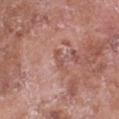Cropped from a whole-body photographic skin survey; the tile spans about 15 mm.
A female patient aged 73 to 77.
From the chest.